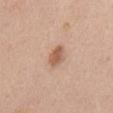Impression:
Captured during whole-body skin photography for melanoma surveillance; the lesion was not biopsied.
Context:
Cropped from a total-body skin-imaging series; the visible field is about 15 mm. On the chest. The patient is a male about 55 years old.